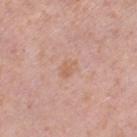  biopsy_status: not biopsied; imaged during a skin examination
  image:
    source: total-body photography crop
    field_of_view_mm: 15
  lighting: white-light
  patient:
    sex: female
    age_approx: 40
  automated_metrics:
    cielab_L: 62
    cielab_a: 21
    cielab_b: 30
    vs_skin_darker_L: 6.0
    vs_skin_contrast_norm: 5.0
    border_irregularity_0_10: 2.5
    peripheral_color_asymmetry: 0.5
  site: left thigh